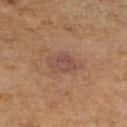Notes:
* biopsy status · imaged on a skin check; not biopsied
* subject · female, aged around 70
* anatomic site · the right lower leg
* illumination · cross-polarized illumination
* lesion size · about 3.5 mm
* image source · ~15 mm tile from a whole-body skin photo